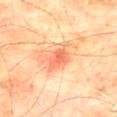No biopsy was performed on this lesion — it was imaged during a full skin examination and was not determined to be concerning. A male subject roughly 75 years of age. Located on the back. A region of skin cropped from a whole-body photographic capture, roughly 15 mm wide.